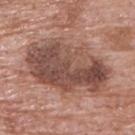Assessment:
Imaged during a routine full-body skin examination; the lesion was not biopsied and no histopathology is available.
Context:
The patient is a male aged 68–72. Cropped from a whole-body photographic skin survey; the tile spans about 15 mm. From the upper back. Imaged with white-light lighting. Longest diameter approximately 10 mm. An algorithmic analysis of the crop reported an area of roughly 45 mm², an outline eccentricity of about 0.8 (0 = round, 1 = elongated), and a shape-asymmetry score of about 0.2 (0 = symmetric). The analysis additionally found border irregularity of about 3 on a 0–10 scale, internal color variation of about 7.5 on a 0–10 scale, and radial color variation of about 2.5.